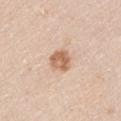Imaged during a routine full-body skin examination; the lesion was not biopsied and no histopathology is available. This is a white-light tile. From the right upper arm. The patient is a male in their mid- to late 40s. This image is a 15 mm lesion crop taken from a total-body photograph.